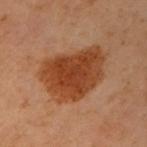workup: catalogued during a skin exam; not biopsied
subject: female, approximately 60 years of age
anatomic site: the left arm
image: ~15 mm tile from a whole-body skin photo